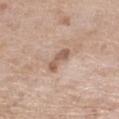follow-up: catalogued during a skin exam; not biopsied
image: ~15 mm crop, total-body skin-cancer survey
automated metrics: an area of roughly 4.5 mm², an outline eccentricity of about 0.9 (0 = round, 1 = elongated), and a shape-asymmetry score of about 0.4 (0 = symmetric); an average lesion color of about L≈58 a*≈18 b*≈28 (CIELAB), a lesion–skin lightness drop of about 11, and a lesion-to-skin contrast of about 7.5 (normalized; higher = more distinct); a within-lesion color-variation index near 1.5/10 and peripheral color asymmetry of about 0.5; a classifier nevus-likeness of about 0/100 and lesion-presence confidence of about 100/100
patient: female, aged around 75
lighting: white-light
anatomic site: the leg
lesion size: ≈3.5 mm